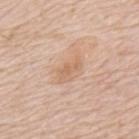* notes: total-body-photography surveillance lesion; no biopsy
* illumination: white-light illumination
* anatomic site: the mid back
* subject: male, about 80 years old
* image source: 15 mm crop, total-body photography